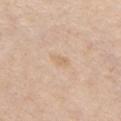| key | value |
|---|---|
| biopsy status | total-body-photography surveillance lesion; no biopsy |
| lesion diameter | ~2.5 mm (longest diameter) |
| lighting | white-light illumination |
| patient | female, in their mid- to late 50s |
| location | the front of the torso |
| image source | ~15 mm crop, total-body skin-cancer survey |
| TBP lesion metrics | border irregularity of about 3 on a 0–10 scale, a within-lesion color-variation index near 0.5/10, and a peripheral color-asymmetry measure near 0; an automated nevus-likeness rating near 0 out of 100 |